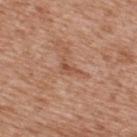Clinical impression:
Part of a total-body skin-imaging series; this lesion was reviewed on a skin check and was not flagged for biopsy.
Background:
A male patient, aged 63–67. Captured under white-light illumination. Cropped from a whole-body photographic skin survey; the tile spans about 15 mm. The lesion is located on the upper back.